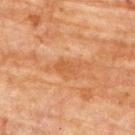Recorded during total-body skin imaging; not selected for excision or biopsy. Cropped from a whole-body photographic skin survey; the tile spans about 15 mm. On the upper back. Captured under cross-polarized illumination. The subject is a female aged 78 to 82.